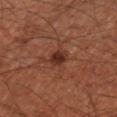Part of a total-body skin-imaging series; this lesion was reviewed on a skin check and was not flagged for biopsy. This is a cross-polarized tile. An algorithmic analysis of the crop reported a nevus-likeness score of about 90/100. The lesion is on the arm. About 2.5 mm across. The patient is a male about 45 years old. A 15 mm close-up tile from a total-body photography series done for melanoma screening.